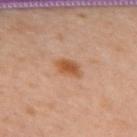biopsy status = catalogued during a skin exam; not biopsied
patient = female, about 40 years old
body site = the upper back
imaging modality = 15 mm crop, total-body photography